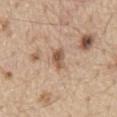Impression:
Recorded during total-body skin imaging; not selected for excision or biopsy.
Clinical summary:
A male patient, aged approximately 70. A 15 mm crop from a total-body photograph taken for skin-cancer surveillance. The lesion-visualizer software estimated a lesion area of about 4 mm², an eccentricity of roughly 0.85, and two-axis asymmetry of about 0.2. The analysis additionally found a border-irregularity index near 2/10, a within-lesion color-variation index near 4/10, and peripheral color asymmetry of about 1. Imaged with white-light lighting. Longest diameter approximately 3 mm. The lesion is on the abdomen.